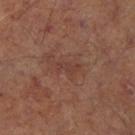| field | value |
|---|---|
| workup | catalogued during a skin exam; not biopsied |
| site | the right lower leg |
| illumination | cross-polarized illumination |
| lesion diameter | ≈3 mm |
| automated metrics | a lesion color around L≈37 a*≈21 b*≈23 in CIELAB, about 5 CIELAB-L* units darker than the surrounding skin, and a normalized border contrast of about 5; a border-irregularity index near 5.5/10, a color-variation rating of about 0/10, and a peripheral color-asymmetry measure near 0; an automated nevus-likeness rating near 0 out of 100 and a lesion-detection confidence of about 100/100 |
| imaging modality | ~15 mm tile from a whole-body skin photo |
| subject | male, about 65 years old |